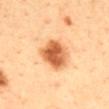Impression: Imaged during a routine full-body skin examination; the lesion was not biopsied and no histopathology is available. Background: This is a cross-polarized tile. A region of skin cropped from a whole-body photographic capture, roughly 15 mm wide. The lesion is located on the mid back. A male patient roughly 40 years of age.